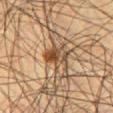  biopsy_status: not biopsied; imaged during a skin examination
  site: leg
  lesion_size:
    long_diameter_mm_approx: 4.0
  image:
    source: total-body photography crop
    field_of_view_mm: 15
  automated_metrics:
    area_mm2_approx: 7.0
    eccentricity: 0.8
    shape_asymmetry: 0.25
    vs_skin_darker_L: 12.0
    vs_skin_contrast_norm: 9.0
    nevus_likeness_0_100: 85
    lesion_detection_confidence_0_100: 100
  patient:
    sex: male
    age_approx: 55